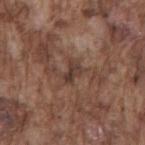Captured during whole-body skin photography for melanoma surveillance; the lesion was not biopsied. Cropped from a whole-body photographic skin survey; the tile spans about 15 mm. The patient is a male roughly 75 years of age. The lesion is located on the mid back. About 2.5 mm across. An algorithmic analysis of the crop reported a lesion area of about 3.5 mm², an eccentricity of roughly 0.8, and two-axis asymmetry of about 0.3. The software also gave a color-variation rating of about 2/10.